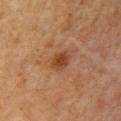Q: Is there a histopathology result?
A: catalogued during a skin exam; not biopsied
Q: Automated lesion metrics?
A: a symmetry-axis asymmetry near 0.15
Q: Patient demographics?
A: female, about 60 years old
Q: Illumination type?
A: cross-polarized
Q: Where on the body is the lesion?
A: the right upper arm
Q: How was this image acquired?
A: total-body-photography crop, ~15 mm field of view